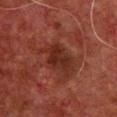Background: The tile uses cross-polarized illumination. Measured at roughly 4.5 mm in maximum diameter. Located on the chest. The subject is a male aged around 60. A close-up tile cropped from a whole-body skin photograph, about 15 mm across.